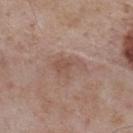<lesion>
<patient>
  <sex>male</sex>
  <age_approx>55</age_approx>
</patient>
<site>upper back</site>
<automated_metrics>
  <cielab_L>52</cielab_L>
  <cielab_a>18</cielab_a>
  <cielab_b>25</cielab_b>
  <vs_skin_darker_L>7.0</vs_skin_darker_L>
  <vs_skin_contrast_norm>5.0</vs_skin_contrast_norm>
  <nevus_likeness_0_100>0</nevus_likeness_0_100>
  <lesion_detection_confidence_0_100>100</lesion_detection_confidence_0_100>
</automated_metrics>
<image>
  <source>total-body photography crop</source>
  <field_of_view_mm>15</field_of_view_mm>
</image>
</lesion>